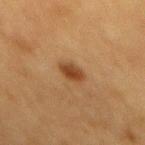No biopsy was performed on this lesion — it was imaged during a full skin examination and was not determined to be concerning. The lesion's longest dimension is about 3 mm. Automated tile analysis of the lesion measured a lesion area of about 5 mm², an eccentricity of roughly 0.7, and a symmetry-axis asymmetry near 0.2. The analysis additionally found a mean CIELAB color near L≈36 a*≈19 b*≈31, roughly 10 lightness units darker than nearby skin, and a normalized lesion–skin contrast near 8.5. It also reported a border-irregularity rating of about 1.5/10, internal color variation of about 3 on a 0–10 scale, and radial color variation of about 1. A female subject approximately 55 years of age. A 15 mm crop from a total-body photograph taken for skin-cancer surveillance. This is a cross-polarized tile. The lesion is located on the mid back.